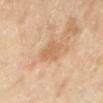Clinical impression:
No biopsy was performed on this lesion — it was imaged during a full skin examination and was not determined to be concerning.
Acquisition and patient details:
Automated image analysis of the tile measured roughly 8 lightness units darker than nearby skin and a normalized border contrast of about 5. And it measured a border-irregularity index near 2.5/10, a color-variation rating of about 2/10, and a peripheral color-asymmetry measure near 0.5. A female patient, about 70 years old. Located on the left lower leg. A roughly 15 mm field-of-view crop from a total-body skin photograph.